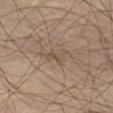<case>
  <biopsy_status>not biopsied; imaged during a skin examination</biopsy_status>
  <automated_metrics>
    <area_mm2_approx>3.5</area_mm2_approx>
    <eccentricity>0.85</eccentricity>
    <shape_asymmetry>0.7</shape_asymmetry>
    <border_irregularity_0_10>7.0</border_irregularity_0_10>
    <nevus_likeness_0_100>0</nevus_likeness_0_100>
    <lesion_detection_confidence_0_100>90</lesion_detection_confidence_0_100>
  </automated_metrics>
  <site>leg</site>
  <image>
    <source>total-body photography crop</source>
    <field_of_view_mm>15</field_of_view_mm>
  </image>
  <patient>
    <sex>male</sex>
    <age_approx>65</age_approx>
  </patient>
  <lesion_size>
    <long_diameter_mm_approx>3.0</long_diameter_mm_approx>
  </lesion_size>
</case>